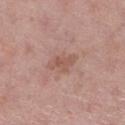Recorded during total-body skin imaging; not selected for excision or biopsy.
A female patient roughly 50 years of age.
A lesion tile, about 15 mm wide, cut from a 3D total-body photograph.
The recorded lesion diameter is about 3.5 mm.
Located on the leg.